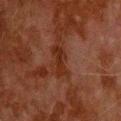  biopsy_status: not biopsied; imaged during a skin examination
  lesion_size:
    long_diameter_mm_approx: 3.5
  patient:
    sex: male
    age_approx: 80
  automated_metrics:
    area_mm2_approx: 4.5
    eccentricity: 0.9
    cielab_L: 22
    cielab_a: 21
    cielab_b: 25
    vs_skin_darker_L: 7.0
    vs_skin_contrast_norm: 8.5
  lighting: cross-polarized
  site: upper back
  image:
    source: total-body photography crop
    field_of_view_mm: 15